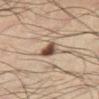Q: Was this lesion biopsied?
A: total-body-photography surveillance lesion; no biopsy
Q: Illumination type?
A: cross-polarized illumination
Q: How large is the lesion?
A: ~3 mm (longest diameter)
Q: What kind of image is this?
A: 15 mm crop, total-body photography
Q: What is the anatomic site?
A: the left lower leg
Q: What did automated image analysis measure?
A: an average lesion color of about L≈44 a*≈15 b*≈25 (CIELAB), about 15 CIELAB-L* units darker than the surrounding skin, and a lesion-to-skin contrast of about 11.5 (normalized; higher = more distinct)
Q: Patient demographics?
A: male, approximately 35 years of age